A 15 mm close-up extracted from a 3D total-body photography capture, this is a cross-polarized tile, Automated image analysis of the tile measured a lesion-to-skin contrast of about 5.5 (normalized; higher = more distinct). And it measured a border-irregularity index near 3/10, the subject is a male in their 60s, longest diameter approximately 3.5 mm, from the chest:
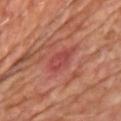Histopathological examination showed a nodular basal cell carcinoma.